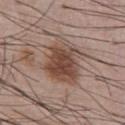Clinical impression: No biopsy was performed on this lesion — it was imaged during a full skin examination and was not determined to be concerning. Context: A 15 mm close-up tile from a total-body photography series done for melanoma screening. The lesion is on the chest. Captured under white-light illumination. A male subject roughly 55 years of age.